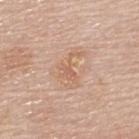Q: Was a biopsy performed?
A: imaged on a skin check; not biopsied
Q: How was the tile lit?
A: white-light
Q: Lesion size?
A: ~4 mm (longest diameter)
Q: What is the anatomic site?
A: the upper back
Q: Patient demographics?
A: male, aged 68–72
Q: What did automated image analysis measure?
A: about 7 CIELAB-L* units darker than the surrounding skin and a lesion-to-skin contrast of about 5 (normalized; higher = more distinct)
Q: What kind of image is this?
A: total-body-photography crop, ~15 mm field of view